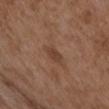workup: catalogued during a skin exam; not biopsied
site: the arm
subject: female, aged 33–37
size: about 2.5 mm
imaging modality: total-body-photography crop, ~15 mm field of view
lighting: white-light
automated metrics: a symmetry-axis asymmetry near 0.25; a mean CIELAB color near L≈41 a*≈19 b*≈28 and about 8 CIELAB-L* units darker than the surrounding skin; a border-irregularity index near 2/10 and radial color variation of about 0.5